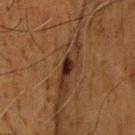follow-up: no biopsy performed (imaged during a skin exam)
automated lesion analysis: a lesion area of about 2.5 mm², an eccentricity of roughly 0.85, and a shape-asymmetry score of about 0.35 (0 = symmetric)
size: ~2.5 mm (longest diameter)
illumination: cross-polarized illumination
image: 15 mm crop, total-body photography
anatomic site: the head or neck
subject: male, in their mid- to late 70s